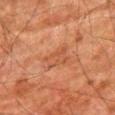biopsy_status: not biopsied; imaged during a skin examination
image:
  source: total-body photography crop
  field_of_view_mm: 15
patient:
  sex: male
  age_approx: 80
lesion_size:
  long_diameter_mm_approx: 3.5
lighting: cross-polarized
site: right thigh
automated_metrics:
  border_irregularity_0_10: 4.0
  color_variation_0_10: 2.5
  peripheral_color_asymmetry: 0.5
  lesion_detection_confidence_0_100: 100